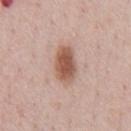| key | value |
|---|---|
| follow-up | catalogued during a skin exam; not biopsied |
| image-analysis metrics | an average lesion color of about L≈56 a*≈21 b*≈28 (CIELAB) and a lesion–skin lightness drop of about 14; a border-irregularity index near 2/10 and a within-lesion color-variation index near 3.5/10; lesion-presence confidence of about 100/100 |
| diameter | about 4.5 mm |
| site | the chest |
| illumination | white-light illumination |
| imaging modality | ~15 mm crop, total-body skin-cancer survey |
| subject | male, approximately 50 years of age |